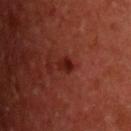{
  "biopsy_status": "not biopsied; imaged during a skin examination",
  "image": {
    "source": "total-body photography crop",
    "field_of_view_mm": 15
  },
  "patient": {
    "sex": "male",
    "age_approx": 60
  },
  "automated_metrics": {
    "area_mm2_approx": 2.5,
    "eccentricity": 0.65,
    "shape_asymmetry": 0.3,
    "cielab_L": 21,
    "cielab_a": 28,
    "cielab_b": 25,
    "vs_skin_darker_L": 8.0,
    "vs_skin_contrast_norm": 9.0
  },
  "site": "upper back",
  "lighting": "cross-polarized",
  "lesion_size": {
    "long_diameter_mm_approx": 2.0
  }
}